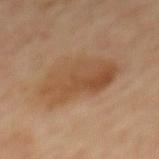biopsy status=imaged on a skin check; not biopsied | lighting=cross-polarized | patient=aged around 65 | anatomic site=the mid back | image=15 mm crop, total-body photography | lesion size=≈7 mm.